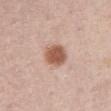| feature | finding |
|---|---|
| follow-up | no biopsy performed (imaged during a skin exam) |
| anatomic site | the abdomen |
| lesion size | about 3.5 mm |
| imaging modality | ~15 mm tile from a whole-body skin photo |
| patient | female, in their mid- to late 60s |
| illumination | white-light illumination |